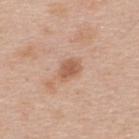Q: Was this lesion biopsied?
A: total-body-photography surveillance lesion; no biopsy
Q: What is the imaging modality?
A: ~15 mm tile from a whole-body skin photo
Q: What are the patient's age and sex?
A: male, approximately 35 years of age
Q: Illumination type?
A: white-light illumination
Q: What did automated image analysis measure?
A: internal color variation of about 1.5 on a 0–10 scale and peripheral color asymmetry of about 0.5
Q: What is the lesion's diameter?
A: about 3 mm
Q: Lesion location?
A: the upper back